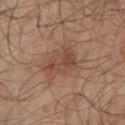Assessment: The lesion was tiled from a total-body skin photograph and was not biopsied. Background: A male subject aged 63 to 67. On the left lower leg. A 15 mm close-up tile from a total-body photography series done for melanoma screening.